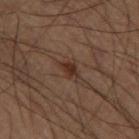<tbp_lesion>
<biopsy_status>not biopsied; imaged during a skin examination</biopsy_status>
<lesion_size>
  <long_diameter_mm_approx>2.5</long_diameter_mm_approx>
</lesion_size>
<automated_metrics>
  <vs_skin_darker_L>7.0</vs_skin_darker_L>
  <vs_skin_contrast_norm>8.0</vs_skin_contrast_norm>
  <nevus_likeness_0_100>50</nevus_likeness_0_100>
  <lesion_detection_confidence_0_100>100</lesion_detection_confidence_0_100>
</automated_metrics>
<patient>
  <sex>male</sex>
  <age_approx>60</age_approx>
</patient>
<lighting>cross-polarized</lighting>
<image>
  <source>total-body photography crop</source>
  <field_of_view_mm>15</field_of_view_mm>
</image>
</tbp_lesion>